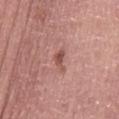Captured during whole-body skin photography for melanoma surveillance; the lesion was not biopsied. The lesion's longest dimension is about 2.5 mm. On the abdomen. Cropped from a whole-body photographic skin survey; the tile spans about 15 mm. A male patient aged 58 to 62.